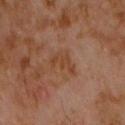A 15 mm close-up tile from a total-body photography series done for melanoma screening. From the chest. A male subject, roughly 60 years of age. The lesion's longest dimension is about 3.5 mm.